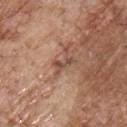Located on the chest.
Automated tile analysis of the lesion measured an area of roughly 2 mm², a shape eccentricity near 0.95, and a shape-asymmetry score of about 0.5 (0 = symmetric). The analysis additionally found a normalized border contrast of about 7.5.
Cropped from a total-body skin-imaging series; the visible field is about 15 mm.
A male subject aged 68–72.
About 2.5 mm across.
The tile uses white-light illumination.